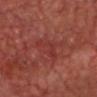No biopsy was performed on this lesion — it was imaged during a full skin examination and was not determined to be concerning. Approximately 4.5 mm at its widest. A lesion tile, about 15 mm wide, cut from a 3D total-body photograph. The lesion is located on the head or neck. A male subject aged around 60. Imaged with cross-polarized lighting.